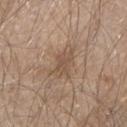Captured during whole-body skin photography for melanoma surveillance; the lesion was not biopsied. On the arm. The lesion-visualizer software estimated a lesion color around L≈52 a*≈15 b*≈29 in CIELAB, roughly 7 lightness units darker than nearby skin, and a lesion-to-skin contrast of about 5.5 (normalized; higher = more distinct). The software also gave a border-irregularity index near 5/10 and internal color variation of about 2 on a 0–10 scale. The tile uses white-light illumination. A male patient, in their mid- to late 60s. The lesion's longest dimension is about 4 mm. A 15 mm close-up extracted from a 3D total-body photography capture.